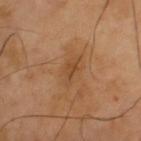Recorded during total-body skin imaging; not selected for excision or biopsy.
The recorded lesion diameter is about 3 mm.
Cropped from a whole-body photographic skin survey; the tile spans about 15 mm.
From the upper back.
A male patient aged 38–42.